The lesion's longest dimension is about 6 mm. Cropped from a whole-body photographic skin survey; the tile spans about 15 mm. From the left upper arm. A female subject in their 40s. Imaged with white-light lighting:
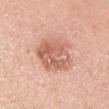Conclusion:
The biopsy diagnosis was a compound melanocytic nevus.